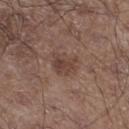No biopsy was performed on this lesion — it was imaged during a full skin examination and was not determined to be concerning. A roughly 15 mm field-of-view crop from a total-body skin photograph. The patient is a male approximately 60 years of age. The tile uses white-light illumination. Approximately 3 mm at its widest. From the left lower leg.